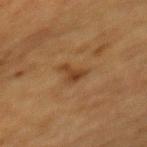| field | value |
|---|---|
| biopsy status | no biopsy performed (imaged during a skin exam) |
| lighting | cross-polarized illumination |
| patient | female, roughly 55 years of age |
| body site | the mid back |
| imaging modality | 15 mm crop, total-body photography |